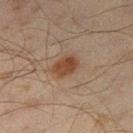No biopsy was performed on this lesion — it was imaged during a full skin examination and was not determined to be concerning.
The recorded lesion diameter is about 3.5 mm.
A male subject roughly 45 years of age.
A lesion tile, about 15 mm wide, cut from a 3D total-body photograph.
Captured under cross-polarized illumination.
The lesion is located on the right thigh.